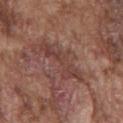  biopsy_status: not biopsied; imaged during a skin examination
  image:
    source: total-body photography crop
    field_of_view_mm: 15
  lighting: white-light
  site: chest
  patient:
    sex: male
    age_approx: 75
  lesion_size:
    long_diameter_mm_approx: 6.5